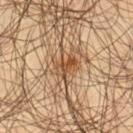* biopsy status · no biopsy performed (imaged during a skin exam)
* illumination · cross-polarized
* acquisition · ~15 mm crop, total-body skin-cancer survey
* lesion size · about 3 mm
* anatomic site · the left thigh
* subject · male, aged 43 to 47